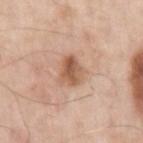Image and clinical context:
An algorithmic analysis of the crop reported a lesion area of about 7.5 mm² and an eccentricity of roughly 0.45. The software also gave a mean CIELAB color near L≈57 a*≈21 b*≈32 and a normalized border contrast of about 8. The analysis additionally found a border-irregularity rating of about 2.5/10 and a color-variation rating of about 6/10. On the left upper arm. A close-up tile cropped from a whole-body skin photograph, about 15 mm across. The recorded lesion diameter is about 3.5 mm. A male patient aged around 55.